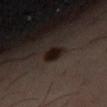automated metrics: a color-variation rating of about 2/10 and radial color variation of about 0.5; site: the abdomen; patient: male, roughly 50 years of age; acquisition: total-body-photography crop, ~15 mm field of view.